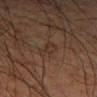<case>
  <biopsy_status>not biopsied; imaged during a skin examination</biopsy_status>
  <patient>
    <sex>male</sex>
    <age_approx>50</age_approx>
  </patient>
  <image>
    <source>total-body photography crop</source>
    <field_of_view_mm>15</field_of_view_mm>
  </image>
  <lighting>cross-polarized</lighting>
  <site>right lower leg</site>
</case>